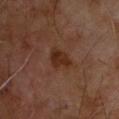Assessment:
Imaged during a routine full-body skin examination; the lesion was not biopsied and no histopathology is available.
Image and clinical context:
A 15 mm crop from a total-body photograph taken for skin-cancer surveillance. The lesion is located on the upper back. Imaged with cross-polarized lighting. Longest diameter approximately 3.5 mm. A male subject, aged 53 to 57.